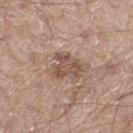Part of a total-body skin-imaging series; this lesion was reviewed on a skin check and was not flagged for biopsy.
A male subject, aged around 65.
On the left thigh.
A roughly 15 mm field-of-view crop from a total-body skin photograph.
Approximately 3.5 mm at its widest.
The tile uses white-light illumination.
An algorithmic analysis of the crop reported an area of roughly 7.5 mm² and a shape-asymmetry score of about 0.45 (0 = symmetric). The software also gave border irregularity of about 5 on a 0–10 scale, internal color variation of about 3.5 on a 0–10 scale, and radial color variation of about 1.